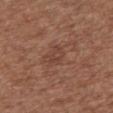<lesion>
  <biopsy_status>not biopsied; imaged during a skin examination</biopsy_status>
  <patient>
    <sex>female</sex>
    <age_approx>75</age_approx>
  </patient>
  <lighting>white-light</lighting>
  <site>front of the torso</site>
  <image>
    <source>total-body photography crop</source>
    <field_of_view_mm>15</field_of_view_mm>
  </image>
  <automated_metrics>
    <nevus_likeness_0_100>0</nevus_likeness_0_100>
    <lesion_detection_confidence_0_100>100</lesion_detection_confidence_0_100>
  </automated_metrics>
</lesion>